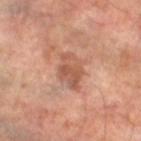workup: imaged on a skin check; not biopsied
image: 15 mm crop, total-body photography
site: the leg
subject: male, in their mid- to late 60s